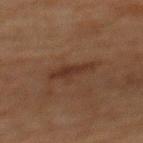Assessment: The lesion was photographed on a routine skin check and not biopsied; there is no pathology result. Context: About 4 mm across. Imaged with cross-polarized lighting. From the mid back. A region of skin cropped from a whole-body photographic capture, roughly 15 mm wide. A female patient, about 60 years old.